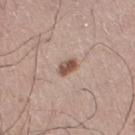Case summary:
– biopsy status: catalogued during a skin exam; not biopsied